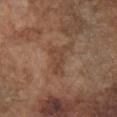The lesion is located on the front of the torso. A close-up tile cropped from a whole-body skin photograph, about 15 mm across. Automated tile analysis of the lesion measured a lesion area of about 7 mm², a shape eccentricity near 0.8, and a symmetry-axis asymmetry near 0.55. The analysis additionally found an average lesion color of about L≈43 a*≈19 b*≈28 (CIELAB) and a lesion-to-skin contrast of about 5.5 (normalized; higher = more distinct). It also reported border irregularity of about 6.5 on a 0–10 scale. Longest diameter approximately 4 mm. Captured under white-light illumination. A male patient, about 75 years old.